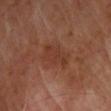Impression: Recorded during total-body skin imaging; not selected for excision or biopsy. Context: The patient is a male approximately 65 years of age. Located on the chest. Measured at roughly 4.5 mm in maximum diameter. A 15 mm crop from a total-body photograph taken for skin-cancer surveillance. The total-body-photography lesion software estimated a lesion color around L≈35 a*≈23 b*≈27 in CIELAB and a lesion-to-skin contrast of about 5.5 (normalized; higher = more distinct). The analysis additionally found a border-irregularity index near 3.5/10, a within-lesion color-variation index near 1.5/10, and peripheral color asymmetry of about 0.5. The software also gave a classifier nevus-likeness of about 0/100 and a lesion-detection confidence of about 100/100. Captured under cross-polarized illumination.